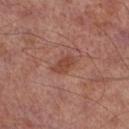This lesion was catalogued during total-body skin photography and was not selected for biopsy.
A male patient in their mid- to late 60s.
This is a cross-polarized tile.
From the right lower leg.
A 15 mm crop from a total-body photograph taken for skin-cancer surveillance.
Automated tile analysis of the lesion measured an eccentricity of roughly 0.75. It also reported a lesion color around L≈42 a*≈23 b*≈27 in CIELAB, about 8 CIELAB-L* units darker than the surrounding skin, and a normalized lesion–skin contrast near 7.
Measured at roughly 3 mm in maximum diameter.